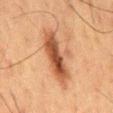notes: no biopsy performed (imaged during a skin exam)
tile lighting: cross-polarized
automated metrics: a shape eccentricity near 0.9 and a shape-asymmetry score of about 0.3 (0 = symmetric); a mean CIELAB color near L≈42 a*≈20 b*≈30, a lesion–skin lightness drop of about 12, and a normalized lesion–skin contrast near 9.5; border irregularity of about 5 on a 0–10 scale and internal color variation of about 5.5 on a 0–10 scale
body site: the back
patient: male, roughly 60 years of age
size: ~7 mm (longest diameter)
image source: ~15 mm crop, total-body skin-cancer survey